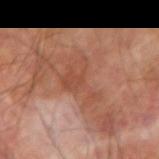Acquisition and patient details: From the left forearm. Imaged with cross-polarized lighting. The patient is a male aged 68 to 72. This image is a 15 mm lesion crop taken from a total-body photograph.